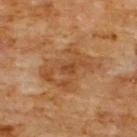Captured during whole-body skin photography for melanoma surveillance; the lesion was not biopsied.
A male patient aged approximately 60.
An algorithmic analysis of the crop reported two-axis asymmetry of about 0.3. It also reported an average lesion color of about L≈47 a*≈21 b*≈36 (CIELAB), roughly 7 lightness units darker than nearby skin, and a normalized lesion–skin contrast near 6.
A 15 mm close-up extracted from a 3D total-body photography capture.
The tile uses cross-polarized illumination.
On the front of the torso.
The lesion's longest dimension is about 6.5 mm.